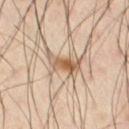The lesion was photographed on a routine skin check and not biopsied; there is no pathology result. The tile uses cross-polarized illumination. A male patient, in their mid-50s. The lesion is located on the right thigh. About 3.5 mm across. A 15 mm close-up tile from a total-body photography series done for melanoma screening.